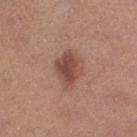notes=imaged on a skin check; not biopsied
site=the right thigh
image source=~15 mm crop, total-body skin-cancer survey
subject=female, aged 38 to 42